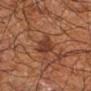biopsy status=catalogued during a skin exam; not biopsied
image=total-body-photography crop, ~15 mm field of view
location=the right upper arm
subject=male, approximately 65 years of age